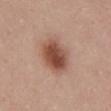Background:
A female patient roughly 25 years of age. A 15 mm close-up tile from a total-body photography series done for melanoma screening. On the mid back.